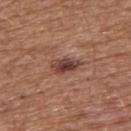From the upper back. Automated image analysis of the tile measured a classifier nevus-likeness of about 80/100 and lesion-presence confidence of about 100/100. Cropped from a whole-body photographic skin survey; the tile spans about 15 mm. The lesion's longest dimension is about 3.5 mm. The patient is a male in their mid- to late 70s.